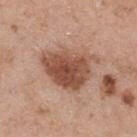Acquisition and patient details: Longest diameter approximately 6.5 mm. A male subject, roughly 55 years of age. Imaged with white-light lighting. The lesion is located on the upper back. A roughly 15 mm field-of-view crop from a total-body skin photograph.